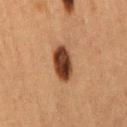Case summary:
- follow-up — total-body-photography surveillance lesion; no biopsy
- imaging modality — ~15 mm crop, total-body skin-cancer survey
- patient — female, approximately 40 years of age
- size — about 4.5 mm
- anatomic site — the lower back
- tile lighting — cross-polarized illumination